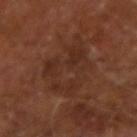Recorded during total-body skin imaging; not selected for excision or biopsy.
A male subject, in their mid- to late 60s.
The lesion is on the right forearm.
A lesion tile, about 15 mm wide, cut from a 3D total-body photograph.